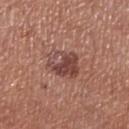Q: Is there a histopathology result?
A: no biopsy performed (imaged during a skin exam)